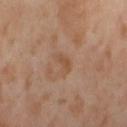{"biopsy_status": "not biopsied; imaged during a skin examination", "lighting": "cross-polarized", "lesion_size": {"long_diameter_mm_approx": 3.0}, "site": "right thigh", "image": {"source": "total-body photography crop", "field_of_view_mm": 15}, "patient": {"sex": "female", "age_approx": 55}}